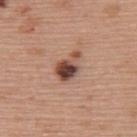No biopsy was performed on this lesion — it was imaged during a full skin examination and was not determined to be concerning.
A female patient about 60 years old.
A region of skin cropped from a whole-body photographic capture, roughly 15 mm wide.
Automated image analysis of the tile measured an eccentricity of roughly 0.75 and two-axis asymmetry of about 0.35. The software also gave a mean CIELAB color near L≈46 a*≈21 b*≈26 and a normalized border contrast of about 12. And it measured an automated nevus-likeness rating near 80 out of 100 and a lesion-detection confidence of about 100/100.
Imaged with white-light lighting.
Longest diameter approximately 4 mm.
The lesion is on the upper back.